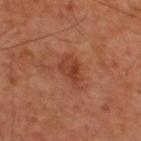Clinical impression: This lesion was catalogued during total-body skin photography and was not selected for biopsy. Context: A male patient aged around 60. Longest diameter approximately 3.5 mm. A lesion tile, about 15 mm wide, cut from a 3D total-body photograph. The tile uses cross-polarized illumination. Located on the back. Automated image analysis of the tile measured a border-irregularity index near 3/10 and a within-lesion color-variation index near 4.5/10.